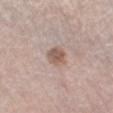| feature | finding |
|---|---|
| follow-up | imaged on a skin check; not biopsied |
| patient | male, aged approximately 80 |
| site | the left thigh |
| image-analysis metrics | an automated nevus-likeness rating near 75 out of 100 and a lesion-detection confidence of about 100/100 |
| acquisition | ~15 mm tile from a whole-body skin photo |
| lighting | white-light illumination |